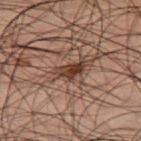This lesion was catalogued during total-body skin photography and was not selected for biopsy. Captured under cross-polarized illumination. The lesion is located on the right thigh. A male subject aged 48–52. A 15 mm crop from a total-body photograph taken for skin-cancer surveillance. Measured at roughly 3 mm in maximum diameter. Automated image analysis of the tile measured a shape eccentricity near 0.8 and a symmetry-axis asymmetry near 0.4. The software also gave a lesion color around L≈27 a*≈15 b*≈20 in CIELAB, roughly 10 lightness units darker than nearby skin, and a normalized lesion–skin contrast near 10.5. The software also gave border irregularity of about 4 on a 0–10 scale, a color-variation rating of about 5.5/10, and radial color variation of about 2.